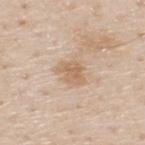Q: Was this lesion biopsied?
A: imaged on a skin check; not biopsied
Q: Illumination type?
A: white-light illumination
Q: What kind of image is this?
A: ~15 mm tile from a whole-body skin photo
Q: Automated lesion metrics?
A: a footprint of about 6.5 mm², a shape eccentricity near 0.65, and a shape-asymmetry score of about 0.25 (0 = symmetric); a border-irregularity rating of about 2.5/10 and radial color variation of about 1; lesion-presence confidence of about 100/100
Q: What are the patient's age and sex?
A: male, in their 80s
Q: Lesion location?
A: the upper back